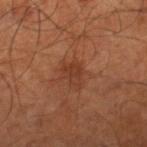notes: imaged on a skin check; not biopsied
lesion diameter: about 3 mm
automated lesion analysis: a lesion area of about 4.5 mm², an eccentricity of roughly 0.7, and a shape-asymmetry score of about 0.4 (0 = symmetric); a mean CIELAB color near L≈37 a*≈24 b*≈31, about 7 CIELAB-L* units darker than the surrounding skin, and a normalized border contrast of about 6; a border-irregularity rating of about 4/10, a color-variation rating of about 1.5/10, and a peripheral color-asymmetry measure near 0.5
acquisition: ~15 mm crop, total-body skin-cancer survey
patient: male, aged 58–62
location: the right lower leg